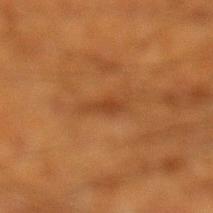Assessment:
No biopsy was performed on this lesion — it was imaged during a full skin examination and was not determined to be concerning.
Clinical summary:
On the left lower leg. Cropped from a whole-body photographic skin survey; the tile spans about 15 mm. A male subject, aged approximately 60.